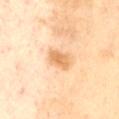Captured during whole-body skin photography for melanoma surveillance; the lesion was not biopsied. An algorithmic analysis of the crop reported about 11 CIELAB-L* units darker than the surrounding skin and a normalized border contrast of about 7.5. A male patient, roughly 70 years of age. Longest diameter approximately 3.5 mm. A 15 mm crop from a total-body photograph taken for skin-cancer surveillance. The tile uses cross-polarized illumination. From the front of the torso.